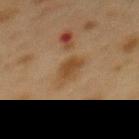  biopsy_status: not biopsied; imaged during a skin examination
  lighting: cross-polarized
  automated_metrics:
    area_mm2_approx: 6.5
    eccentricity: 0.85
    shape_asymmetry: 0.25
    cielab_L: 38
    cielab_a: 15
    cielab_b: 30
    vs_skin_darker_L: 7.0
    vs_skin_contrast_norm: 7.0
  patient:
    sex: female
    age_approx: 40
  image:
    source: total-body photography crop
    field_of_view_mm: 15
  site: mid back